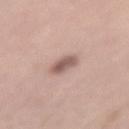workup = imaged on a skin check; not biopsied
size = ~3 mm (longest diameter)
subject = female, roughly 40 years of age
body site = the lower back
acquisition = ~15 mm crop, total-body skin-cancer survey
illumination = white-light illumination